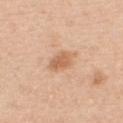workup — total-body-photography surveillance lesion; no biopsy
image source — 15 mm crop, total-body photography
anatomic site — the chest
tile lighting — white-light
patient — male, aged 48–52
diameter — ~3.5 mm (longest diameter)